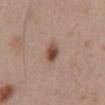Recorded during total-body skin imaging; not selected for excision or biopsy.